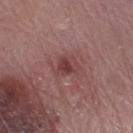No biopsy was performed on this lesion — it was imaged during a full skin examination and was not determined to be concerning. Located on the right lower leg. A female subject, approximately 70 years of age. An algorithmic analysis of the crop reported an average lesion color of about L≈41 a*≈24 b*≈20 (CIELAB), about 8 CIELAB-L* units darker than the surrounding skin, and a normalized border contrast of about 7. And it measured a border-irregularity rating of about 3.5/10, a within-lesion color-variation index near 3.5/10, and radial color variation of about 1. A lesion tile, about 15 mm wide, cut from a 3D total-body photograph. The recorded lesion diameter is about 3 mm.